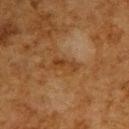The lesion was tiled from a total-body skin photograph and was not biopsied.
The patient is a male aged 58 to 62.
The total-body-photography lesion software estimated a lesion area of about 5 mm² and two-axis asymmetry of about 0.3. The analysis additionally found a nevus-likeness score of about 0/100.
Cropped from a whole-body photographic skin survey; the tile spans about 15 mm.
From the upper back.